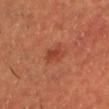biopsy_status: not biopsied; imaged during a skin examination
image:
  source: total-body photography crop
  field_of_view_mm: 15
patient:
  sex: male
  age_approx: 60
lesion_size:
  long_diameter_mm_approx: 2.5
lighting: cross-polarized
site: chest
automated_metrics:
  color_variation_0_10: 3.0
  peripheral_color_asymmetry: 1.0
  lesion_detection_confidence_0_100: 100